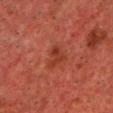Assessment:
No biopsy was performed on this lesion — it was imaged during a full skin examination and was not determined to be concerning.
Acquisition and patient details:
This image is a 15 mm lesion crop taken from a total-body photograph. On the chest. The patient is a male in their 50s.